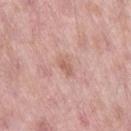Q: Is there a histopathology result?
A: no biopsy performed (imaged during a skin exam)
Q: What is the imaging modality?
A: 15 mm crop, total-body photography
Q: What is the anatomic site?
A: the leg
Q: What is the lesion's diameter?
A: ≈2.5 mm
Q: Patient demographics?
A: male, roughly 55 years of age